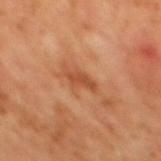Clinical impression:
Recorded during total-body skin imaging; not selected for excision or biopsy.
Clinical summary:
Measured at roughly 3 mm in maximum diameter. A close-up tile cropped from a whole-body skin photograph, about 15 mm across. A male patient, approximately 65 years of age. The tile uses cross-polarized illumination. The lesion is located on the mid back.